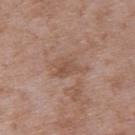Q: What is the lesion's diameter?
A: ≈4 mm
Q: Automated lesion metrics?
A: a lesion area of about 6.5 mm², an eccentricity of roughly 0.8, and two-axis asymmetry of about 0.4; an average lesion color of about L≈51 a*≈19 b*≈28 (CIELAB), roughly 7 lightness units darker than nearby skin, and a normalized lesion–skin contrast near 5.5; a border-irregularity rating of about 5/10, a color-variation rating of about 3/10, and radial color variation of about 1
Q: Patient demographics?
A: male, aged 48–52
Q: What is the anatomic site?
A: the upper back
Q: What is the imaging modality?
A: ~15 mm crop, total-body skin-cancer survey